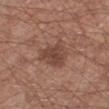Assessment: The lesion was tiled from a total-body skin photograph and was not biopsied. Background: The recorded lesion diameter is about 4.5 mm. A region of skin cropped from a whole-body photographic capture, roughly 15 mm wide. Imaged with white-light lighting. Located on the left lower leg. A male patient, about 65 years old. The lesion-visualizer software estimated a footprint of about 11 mm², a shape eccentricity near 0.7, and two-axis asymmetry of about 0.3. The analysis additionally found a classifier nevus-likeness of about 45/100 and a lesion-detection confidence of about 100/100.